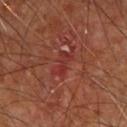Q: How large is the lesion?
A: ≈3 mm
Q: What is the imaging modality?
A: ~15 mm crop, total-body skin-cancer survey
Q: Automated lesion metrics?
A: a footprint of about 3.5 mm² and an outline eccentricity of about 0.9 (0 = round, 1 = elongated); a lesion color around L≈34 a*≈31 b*≈27 in CIELAB, a lesion–skin lightness drop of about 6, and a normalized lesion–skin contrast near 5.5
Q: Lesion location?
A: the arm
Q: How was the tile lit?
A: cross-polarized illumination
Q: Patient demographics?
A: approximately 65 years of age